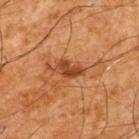Captured during whole-body skin photography for melanoma surveillance; the lesion was not biopsied.
A 15 mm close-up tile from a total-body photography series done for melanoma screening.
Approximately 3.5 mm at its widest.
On the upper back.
The patient is a male aged 63 to 67.